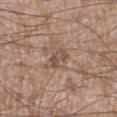follow-up: imaged on a skin check; not biopsied | subject: male, in their 60s | site: the left lower leg | automated lesion analysis: a classifier nevus-likeness of about 0/100 and lesion-presence confidence of about 90/100 | image: ~15 mm crop, total-body skin-cancer survey.